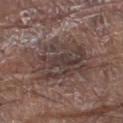Assessment: This lesion was catalogued during total-body skin photography and was not selected for biopsy. Acquisition and patient details: The lesion is located on the leg. A 15 mm close-up tile from a total-body photography series done for melanoma screening. A male patient, aged around 80.